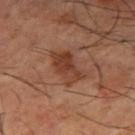No biopsy was performed on this lesion — it was imaged during a full skin examination and was not determined to be concerning. Automated tile analysis of the lesion measured a shape eccentricity near 0.8 and two-axis asymmetry of about 0.3. The analysis additionally found a border-irregularity rating of about 3/10 and a within-lesion color-variation index near 4.5/10. It also reported a lesion-detection confidence of about 100/100. The recorded lesion diameter is about 4.5 mm. This image is a 15 mm lesion crop taken from a total-body photograph. Located on the right thigh. The subject is a male aged 63–67.